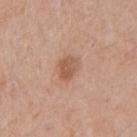  automated_metrics:
    area_mm2_approx: 5.5
    eccentricity: 0.55
    nevus_likeness_0_100: 75
  patient:
    sex: male
    age_approx: 60
  site: right upper arm
  image:
    source: total-body photography crop
    field_of_view_mm: 15
  lighting: white-light